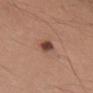workup: imaged on a skin check; not biopsied
size: ~3 mm (longest diameter)
patient: male, aged 23–27
site: the left upper arm
image: total-body-photography crop, ~15 mm field of view
illumination: white-light
image-analysis metrics: an outline eccentricity of about 0.9 (0 = round, 1 = elongated) and a shape-asymmetry score of about 0.25 (0 = symmetric); a border-irregularity index near 2.5/10